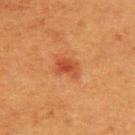The lesion was photographed on a routine skin check and not biopsied; there is no pathology result.
Captured under cross-polarized illumination.
An algorithmic analysis of the crop reported internal color variation of about 3.5 on a 0–10 scale and a peripheral color-asymmetry measure near 1. It also reported a classifier nevus-likeness of about 80/100 and a detector confidence of about 100 out of 100 that the crop contains a lesion.
Cropped from a total-body skin-imaging series; the visible field is about 15 mm.
The lesion is located on the back.
A female patient, aged 38 to 42.
Measured at roughly 3 mm in maximum diameter.